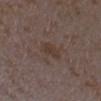Part of a total-body skin-imaging series; this lesion was reviewed on a skin check and was not flagged for biopsy. The lesion is on the left forearm. The patient is a female aged around 35. A 15 mm close-up extracted from a 3D total-body photography capture. The tile uses white-light illumination.